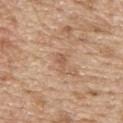{"biopsy_status": "not biopsied; imaged during a skin examination", "automated_metrics": {"cielab_L": 57, "cielab_a": 19, "cielab_b": 32, "vs_skin_darker_L": 8.0, "vs_skin_contrast_norm": 5.5, "lesion_detection_confidence_0_100": 100}, "lighting": "white-light", "lesion_size": {"long_diameter_mm_approx": 2.5}, "image": {"source": "total-body photography crop", "field_of_view_mm": 15}, "site": "upper back", "patient": {"sex": "male", "age_approx": 70}}